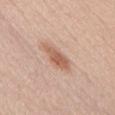workup: catalogued during a skin exam; not biopsied | subject: female, aged around 40 | anatomic site: the front of the torso | imaging modality: ~15 mm crop, total-body skin-cancer survey.